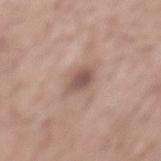Q: Is there a histopathology result?
A: no biopsy performed (imaged during a skin exam)
Q: Who is the patient?
A: male, aged around 60
Q: How was this image acquired?
A: ~15 mm crop, total-body skin-cancer survey
Q: Where on the body is the lesion?
A: the back